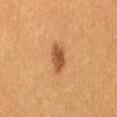Q: Is there a histopathology result?
A: imaged on a skin check; not biopsied
Q: What is the anatomic site?
A: the leg
Q: Illumination type?
A: cross-polarized illumination
Q: How large is the lesion?
A: about 3.5 mm
Q: What is the imaging modality?
A: ~15 mm tile from a whole-body skin photo
Q: Who is the patient?
A: female, aged approximately 40